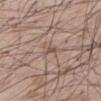Captured during whole-body skin photography for melanoma surveillance; the lesion was not biopsied.
The lesion is located on the back.
A roughly 15 mm field-of-view crop from a total-body skin photograph.
A male subject aged 63 to 67.
This is a white-light tile.
The recorded lesion diameter is about 2.5 mm.
An algorithmic analysis of the crop reported a lesion color around L≈52 a*≈16 b*≈23 in CIELAB and roughly 8 lightness units darker than nearby skin. The analysis additionally found internal color variation of about 0 on a 0–10 scale. The analysis additionally found a classifier nevus-likeness of about 5/100.